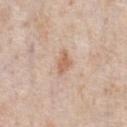notes: no biopsy performed (imaged during a skin exam)
lighting: white-light illumination
patient: male, aged 58 to 62
image source: 15 mm crop, total-body photography
diameter: ~3 mm (longest diameter)
location: the chest